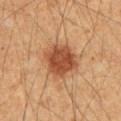Case summary:
– automated metrics: an area of roughly 13 mm² and an outline eccentricity of about 0.3 (0 = round, 1 = elongated); an average lesion color of about L≈44 a*≈23 b*≈33 (CIELAB) and a lesion-to-skin contrast of about 9.5 (normalized; higher = more distinct); a border-irregularity index near 2/10 and peripheral color asymmetry of about 1; a nevus-likeness score of about 100/100 and lesion-presence confidence of about 100/100
– site: the mid back
– tile lighting: cross-polarized
– image source: ~15 mm tile from a whole-body skin photo
– subject: male, aged around 60
– diameter: ≈4 mm